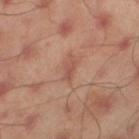The subject is a male aged approximately 45.
A region of skin cropped from a whole-body photographic capture, roughly 15 mm wide.
Located on the leg.
Automated image analysis of the tile measured a mean CIELAB color near L≈51 a*≈23 b*≈27, about 8 CIELAB-L* units darker than the surrounding skin, and a normalized border contrast of about 5.5. And it measured an automated nevus-likeness rating near 0 out of 100.
The tile uses cross-polarized illumination.